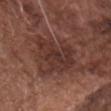Clinical impression: This lesion was catalogued during total-body skin photography and was not selected for biopsy. Background: From the front of the torso. Longest diameter approximately 5.5 mm. Automated image analysis of the tile measured an area of roughly 19 mm² and a shape-asymmetry score of about 0.35 (0 = symmetric). The software also gave border irregularity of about 5 on a 0–10 scale, a within-lesion color-variation index near 3.5/10, and peripheral color asymmetry of about 1. The software also gave a detector confidence of about 100 out of 100 that the crop contains a lesion. This is a white-light tile. The patient is a male aged approximately 75. A 15 mm crop from a total-body photograph taken for skin-cancer surveillance.